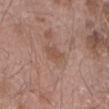Part of a total-body skin-imaging series; this lesion was reviewed on a skin check and was not flagged for biopsy. A male patient about 55 years old. Automated image analysis of the tile measured a lesion area of about 4.5 mm², an outline eccentricity of about 0.8 (0 = round, 1 = elongated), and a symmetry-axis asymmetry near 0.3. And it measured a lesion color around L≈51 a*≈19 b*≈27 in CIELAB, roughly 7 lightness units darker than nearby skin, and a normalized border contrast of about 5.5. The analysis additionally found a border-irregularity rating of about 3/10, a within-lesion color-variation index near 1.5/10, and radial color variation of about 0.5. The analysis additionally found an automated nevus-likeness rating near 0 out of 100. The lesion is located on the lower back. The lesion's longest dimension is about 3 mm. This is a white-light tile. This image is a 15 mm lesion crop taken from a total-body photograph.